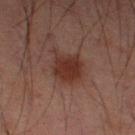Clinical summary: Located on the right thigh. The tile uses cross-polarized illumination. The lesion's longest dimension is about 4.5 mm. The patient is a male about 60 years old. Cropped from a whole-body photographic skin survey; the tile spans about 15 mm.